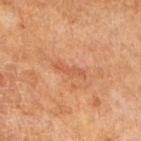Q: Was this lesion biopsied?
A: total-body-photography surveillance lesion; no biopsy
Q: What is the imaging modality?
A: ~15 mm crop, total-body skin-cancer survey
Q: Who is the patient?
A: male, aged around 65
Q: What lighting was used for the tile?
A: cross-polarized illumination
Q: What did automated image analysis measure?
A: about 7 CIELAB-L* units darker than the surrounding skin; peripheral color asymmetry of about 0
Q: Lesion size?
A: ≈3.5 mm